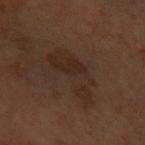Q: What kind of image is this?
A: 15 mm crop, total-body photography
Q: Illumination type?
A: cross-polarized
Q: Lesion location?
A: the front of the torso
Q: Who is the patient?
A: female, approximately 60 years of age
Q: Lesion size?
A: about 6.5 mm
Q: Automated lesion metrics?
A: a footprint of about 14 mm² and a shape eccentricity near 0.85; a border-irregularity rating of about 7.5/10, a color-variation rating of about 1.5/10, and radial color variation of about 0.5